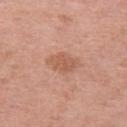Case summary:
– notes: total-body-photography surveillance lesion; no biopsy
– illumination: white-light
– image: ~15 mm tile from a whole-body skin photo
– diameter: ~3.5 mm (longest diameter)
– patient: female, aged approximately 45
– anatomic site: the left upper arm